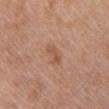Q: Is there a histopathology result?
A: no biopsy performed (imaged during a skin exam)
Q: How large is the lesion?
A: ~2.5 mm (longest diameter)
Q: What did automated image analysis measure?
A: a footprint of about 3.5 mm² and a shape-asymmetry score of about 0.45 (0 = symmetric); an average lesion color of about L≈54 a*≈21 b*≈31 (CIELAB); a classifier nevus-likeness of about 10/100 and a detector confidence of about 100 out of 100 that the crop contains a lesion
Q: Lesion location?
A: the chest
Q: How was this image acquired?
A: 15 mm crop, total-body photography
Q: Patient demographics?
A: male, aged around 65
Q: What lighting was used for the tile?
A: white-light